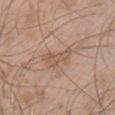| key | value |
|---|---|
| follow-up | total-body-photography surveillance lesion; no biopsy |
| image | ~15 mm crop, total-body skin-cancer survey |
| automated lesion analysis | a lesion color around L≈56 a*≈17 b*≈29 in CIELAB and a normalized lesion–skin contrast near 5.5 |
| site | the front of the torso |
| patient | male, aged 28 to 32 |
| diameter | about 4.5 mm |
| lighting | white-light illumination |